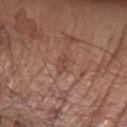Findings:
- notes — catalogued during a skin exam; not biopsied
- location — the arm
- diameter — ~2.5 mm (longest diameter)
- automated metrics — a color-variation rating of about 2.5/10 and radial color variation of about 1; an automated nevus-likeness rating near 0 out of 100 and a detector confidence of about 95 out of 100 that the crop contains a lesion
- subject — male, aged 68 to 72
- imaging modality — ~15 mm crop, total-body skin-cancer survey
- tile lighting — white-light